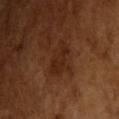Clinical impression: Recorded during total-body skin imaging; not selected for excision or biopsy. Image and clinical context: A male patient aged 63–67. A 15 mm close-up tile from a total-body photography series done for melanoma screening.